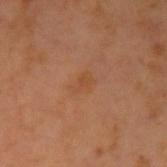workup: total-body-photography surveillance lesion; no biopsy
anatomic site: the left upper arm
image source: ~15 mm crop, total-body skin-cancer survey
subject: male, aged 58–62